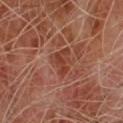Q: Was this lesion biopsied?
A: imaged on a skin check; not biopsied
Q: What is the lesion's diameter?
A: ≈3.5 mm
Q: What is the imaging modality?
A: ~15 mm crop, total-body skin-cancer survey
Q: Illumination type?
A: cross-polarized
Q: Who is the patient?
A: male, aged approximately 65